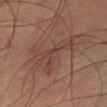{"biopsy_status": "not biopsied; imaged during a skin examination", "patient": {"sex": "male", "age_approx": 60}, "image": {"source": "total-body photography crop", "field_of_view_mm": 15}, "lighting": "cross-polarized"}